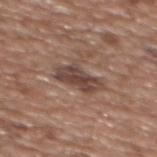<record>
  <biopsy_status>not biopsied; imaged during a skin examination</biopsy_status>
  <image>
    <source>total-body photography crop</source>
    <field_of_view_mm>15</field_of_view_mm>
  </image>
  <site>upper back</site>
  <lighting>white-light</lighting>
  <patient>
    <sex>female</sex>
    <age_approx>75</age_approx>
  </patient>
  <lesion_size>
    <long_diameter_mm_approx>5.0</long_diameter_mm_approx>
  </lesion_size>
  <automated_metrics>
    <cielab_L>43</cielab_L>
    <cielab_a>17</cielab_a>
    <cielab_b>23</cielab_b>
    <vs_skin_darker_L>11.0</vs_skin_darker_L>
    <vs_skin_contrast_norm>9.0</vs_skin_contrast_norm>
  </automated_metrics>
</record>